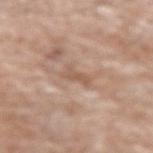Impression: The lesion was tiled from a total-body skin photograph and was not biopsied. Background: The lesion is on the right forearm. Measured at roughly 3 mm in maximum diameter. Automated tile analysis of the lesion measured a lesion color around L≈56 a*≈19 b*≈29 in CIELAB and a lesion–skin lightness drop of about 8. It also reported an automated nevus-likeness rating near 0 out of 100 and a detector confidence of about 60 out of 100 that the crop contains a lesion. A 15 mm close-up tile from a total-body photography series done for melanoma screening. The patient is a male in their 80s.